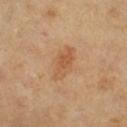The lesion was tiled from a total-body skin photograph and was not biopsied.
The lesion-visualizer software estimated an outline eccentricity of about 0.85 (0 = round, 1 = elongated) and a shape-asymmetry score of about 0.2 (0 = symmetric). The software also gave a border-irregularity index near 2.5/10, a within-lesion color-variation index near 3.5/10, and a peripheral color-asymmetry measure near 1.5. And it measured a nevus-likeness score of about 35/100 and a lesion-detection confidence of about 100/100.
On the leg.
A roughly 15 mm field-of-view crop from a total-body skin photograph.
Longest diameter approximately 4.5 mm.
A female patient, about 60 years old.
Captured under cross-polarized illumination.